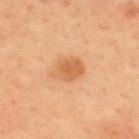follow-up: no biopsy performed (imaged during a skin exam).